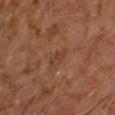Q: What is the imaging modality?
A: ~15 mm crop, total-body skin-cancer survey
Q: Illumination type?
A: cross-polarized illumination
Q: How large is the lesion?
A: ≈3 mm
Q: Patient demographics?
A: male, roughly 60 years of age
Q: Where on the body is the lesion?
A: the chest